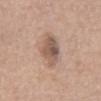Q: Is there a histopathology result?
A: imaged on a skin check; not biopsied